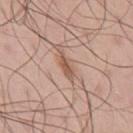Case summary:
* illumination — white-light
* patient — male, about 45 years old
* size — ~3 mm (longest diameter)
* imaging modality — 15 mm crop, total-body photography
* automated metrics — an area of roughly 3.5 mm² and an eccentricity of roughly 0.9; a border-irregularity index near 3.5/10, a within-lesion color-variation index near 1.5/10, and peripheral color asymmetry of about 0.5; a classifier nevus-likeness of about 5/100 and a lesion-detection confidence of about 100/100
* location — the upper back